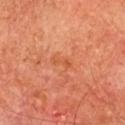{
  "biopsy_status": "not biopsied; imaged during a skin examination",
  "patient": {
    "sex": "male",
    "age_approx": 65
  },
  "site": "chest",
  "image": {
    "source": "total-body photography crop",
    "field_of_view_mm": 15
  }
}